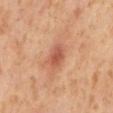The lesion was tiled from a total-body skin photograph and was not biopsied.
The patient is a male in their 60s.
Located on the mid back.
Cropped from a total-body skin-imaging series; the visible field is about 15 mm.
Imaged with cross-polarized lighting.